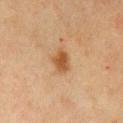Part of a total-body skin-imaging series; this lesion was reviewed on a skin check and was not flagged for biopsy. The lesion-visualizer software estimated a lesion area of about 5 mm² and a symmetry-axis asymmetry near 0.2. About 3 mm across. A female subject aged approximately 50. A close-up tile cropped from a whole-body skin photograph, about 15 mm across. This is a cross-polarized tile. The lesion is on the chest.